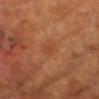Clinical summary: Longest diameter approximately 2.5 mm. The patient is a male approximately 60 years of age. A 15 mm crop from a total-body photograph taken for skin-cancer surveillance. An algorithmic analysis of the crop reported border irregularity of about 1.5 on a 0–10 scale, a color-variation rating of about 1.5/10, and peripheral color asymmetry of about 0.5. This is a cross-polarized tile. The lesion is located on the front of the torso. Conclusion: On biopsy, histopathology showed a skin cancer: nodular basal cell carcinoma.